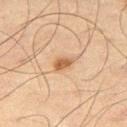patient: male, in their mid- to late 60s
lighting: cross-polarized
lesion diameter: ~3 mm (longest diameter)
TBP lesion metrics: a lesion area of about 4 mm², an eccentricity of roughly 0.8, and a shape-asymmetry score of about 0.25 (0 = symmetric); a mean CIELAB color near L≈49 a*≈18 b*≈31, roughly 10 lightness units darker than nearby skin, and a normalized border contrast of about 8; radial color variation of about 0.5
image: ~15 mm tile from a whole-body skin photo
anatomic site: the right thigh